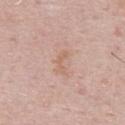Part of a total-body skin-imaging series; this lesion was reviewed on a skin check and was not flagged for biopsy. An algorithmic analysis of the crop reported an average lesion color of about L≈63 a*≈19 b*≈29 (CIELAB), roughly 6 lightness units darker than nearby skin, and a normalized lesion–skin contrast near 5. And it measured an automated nevus-likeness rating near 0 out of 100. A male subject, in their 70s. This image is a 15 mm lesion crop taken from a total-body photograph. Imaged with white-light lighting. The lesion is on the upper back. The lesion's longest dimension is about 3 mm.